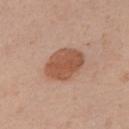– notes — catalogued during a skin exam; not biopsied
– patient — male, in their mid- to late 50s
– acquisition — ~15 mm tile from a whole-body skin photo
– automated metrics — an area of roughly 14 mm² and an outline eccentricity of about 0.65 (0 = round, 1 = elongated); a lesion–skin lightness drop of about 12 and a lesion-to-skin contrast of about 8.5 (normalized; higher = more distinct); a classifier nevus-likeness of about 95/100 and a detector confidence of about 100 out of 100 that the crop contains a lesion
– anatomic site — the chest
– lesion diameter — ~5 mm (longest diameter)
– illumination — white-light